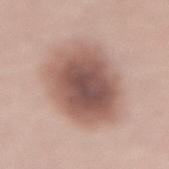Imaged during a routine full-body skin examination; the lesion was not biopsied and no histopathology is available. On the lower back. The lesion's longest dimension is about 8.5 mm. Captured under white-light illumination. A 15 mm close-up extracted from a 3D total-body photography capture. A male subject, roughly 55 years of age.